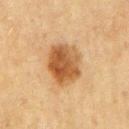Q: Was a biopsy performed?
A: catalogued during a skin exam; not biopsied
Q: Patient demographics?
A: male, in their 70s
Q: How was the tile lit?
A: cross-polarized illumination
Q: How was this image acquired?
A: 15 mm crop, total-body photography
Q: What did automated image analysis measure?
A: an area of roughly 16 mm² and two-axis asymmetry of about 0.15; an average lesion color of about L≈46 a*≈18 b*≈35 (CIELAB), roughly 12 lightness units darker than nearby skin, and a normalized lesion–skin contrast near 9.5; an automated nevus-likeness rating near 100 out of 100 and a lesion-detection confidence of about 100/100
Q: What is the anatomic site?
A: the right upper arm
Q: How large is the lesion?
A: ≈5.5 mm